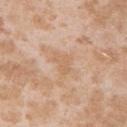Impression:
No biopsy was performed on this lesion — it was imaged during a full skin examination and was not determined to be concerning.